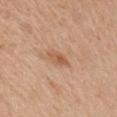Notes:
– biopsy status — imaged on a skin check; not biopsied
– body site — the front of the torso
– acquisition — total-body-photography crop, ~15 mm field of view
– patient — male, about 60 years old